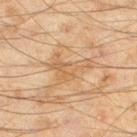biopsy_status: not biopsied; imaged during a skin examination
image:
  source: total-body photography crop
  field_of_view_mm: 15
lighting: cross-polarized
site: left thigh
patient:
  sex: male
  age_approx: 45
lesion_size:
  long_diameter_mm_approx: 4.0